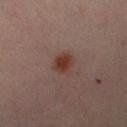follow-up: imaged on a skin check; not biopsied
acquisition: total-body-photography crop, ~15 mm field of view
lighting: cross-polarized illumination
subject: female, aged 38 to 42
lesion diameter: ≈2.5 mm
site: the right lower leg